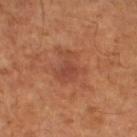| field | value |
|---|---|
| workup | catalogued during a skin exam; not biopsied |
| lesion diameter | ≈4 mm |
| imaging modality | total-body-photography crop, ~15 mm field of view |
| patient | male, aged 63 to 67 |
| tile lighting | cross-polarized illumination |